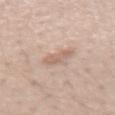Case summary:
* biopsy status · imaged on a skin check; not biopsied
* TBP lesion metrics · peripheral color asymmetry of about 0.5; an automated nevus-likeness rating near 10 out of 100 and lesion-presence confidence of about 100/100
* lesion size · ≈3 mm
* lighting · white-light illumination
* acquisition · ~15 mm crop, total-body skin-cancer survey
* subject · female, about 40 years old
* anatomic site · the right forearm